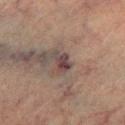The lesion is located on the left lower leg.
A male subject, in their mid- to late 70s.
Cropped from a whole-body photographic skin survey; the tile spans about 15 mm.
Measured at roughly 2.5 mm in maximum diameter.
Captured under cross-polarized illumination.